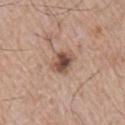follow-up=catalogued during a skin exam; not biopsied
subject=male, roughly 75 years of age
body site=the right upper arm
diameter=about 3 mm
acquisition=15 mm crop, total-body photography
illumination=white-light illumination